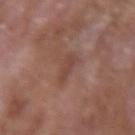acquisition = ~15 mm crop, total-body skin-cancer survey
lesion diameter = ≈2.5 mm
patient = male, roughly 65 years of age
anatomic site = the right forearm
lighting = white-light illumination